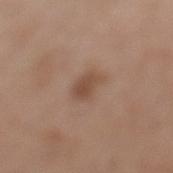Part of a total-body skin-imaging series; this lesion was reviewed on a skin check and was not flagged for biopsy.
Longest diameter approximately 3.5 mm.
Captured under white-light illumination.
Located on the left lower leg.
A male subject, about 70 years old.
A 15 mm close-up tile from a total-body photography series done for melanoma screening.